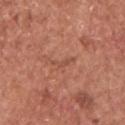  biopsy_status: not biopsied; imaged during a skin examination
  image:
    source: total-body photography crop
    field_of_view_mm: 15
  site: front of the torso
  lesion_size:
    long_diameter_mm_approx: 3.0
  patient:
    sex: male
    age_approx: 75